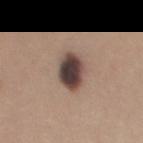Q: Was this lesion biopsied?
A: total-body-photography surveillance lesion; no biopsy
Q: What is the anatomic site?
A: the upper back
Q: How large is the lesion?
A: about 4 mm
Q: What are the patient's age and sex?
A: female, aged approximately 35
Q: How was the tile lit?
A: white-light
Q: How was this image acquired?
A: ~15 mm crop, total-body skin-cancer survey